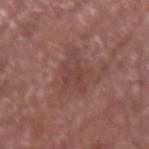Notes:
* workup: no biopsy performed (imaged during a skin exam)